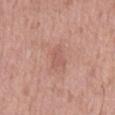The lesion was photographed on a routine skin check and not biopsied; there is no pathology result. The tile uses white-light illumination. On the mid back. A region of skin cropped from a whole-body photographic capture, roughly 15 mm wide. Automated tile analysis of the lesion measured a lesion area of about 4.5 mm² and a shape-asymmetry score of about 0.25 (0 = symmetric). It also reported a border-irregularity index near 3/10 and a color-variation rating of about 1/10. The software also gave lesion-presence confidence of about 100/100. A male patient in their mid-50s.